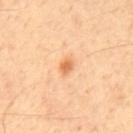Q: Was a biopsy performed?
A: no biopsy performed (imaged during a skin exam)
Q: What is the lesion's diameter?
A: about 2 mm
Q: Lesion location?
A: the mid back
Q: Who is the patient?
A: male, in their mid-50s
Q: What kind of image is this?
A: 15 mm crop, total-body photography
Q: What lighting was used for the tile?
A: cross-polarized illumination